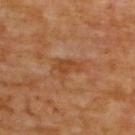Assessment:
No biopsy was performed on this lesion — it was imaged during a full skin examination and was not determined to be concerning.
Clinical summary:
A male subject, aged around 60. Cropped from a whole-body photographic skin survey; the tile spans about 15 mm. Approximately 3.5 mm at its widest. On the upper back. An algorithmic analysis of the crop reported a footprint of about 5.5 mm² and a shape-asymmetry score of about 0.45 (0 = symmetric). The software also gave about 6 CIELAB-L* units darker than the surrounding skin and a normalized border contrast of about 6. It also reported border irregularity of about 5 on a 0–10 scale, internal color variation of about 2 on a 0–10 scale, and a peripheral color-asymmetry measure near 0.5.